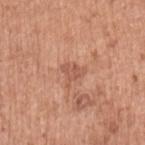Findings:
• notes — total-body-photography surveillance lesion; no biopsy
• diameter — ~3 mm (longest diameter)
• automated lesion analysis — a border-irregularity index near 5/10 and internal color variation of about 1 on a 0–10 scale
• acquisition — 15 mm crop, total-body photography
• anatomic site — the arm
• tile lighting — white-light
• patient — female, aged approximately 50